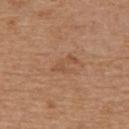lighting = white-light illumination | diameter = ~3 mm (longest diameter) | subject = male, aged 68 to 72 | automated metrics = a shape eccentricity near 0.9 and a symmetry-axis asymmetry near 0.5; a lesion color around L≈51 a*≈21 b*≈34 in CIELAB, a lesion–skin lightness drop of about 6, and a normalized border contrast of about 5 | imaging modality = 15 mm crop, total-body photography | anatomic site = the upper back.